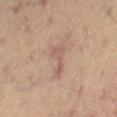Notes:
* TBP lesion metrics — an average lesion color of about L≈50 a*≈17 b*≈22 (CIELAB), a lesion–skin lightness drop of about 7, and a lesion-to-skin contrast of about 5.5 (normalized; higher = more distinct)
* lesion size — ~4.5 mm (longest diameter)
* subject — male, aged approximately 65
* image source — ~15 mm tile from a whole-body skin photo
* illumination — cross-polarized
* body site — the right thigh